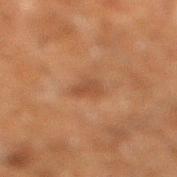Impression: Captured during whole-body skin photography for melanoma surveillance; the lesion was not biopsied. Background: The lesion is on the leg. This image is a 15 mm lesion crop taken from a total-body photograph. The recorded lesion diameter is about 2.5 mm. A male patient, roughly 60 years of age. Imaged with cross-polarized lighting.